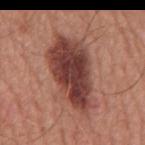workup = catalogued during a skin exam; not biopsied
site = the mid back
subject = male, aged 63 to 67
image = 15 mm crop, total-body photography
size = ≈10 mm
tile lighting = white-light